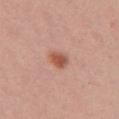Q: Was a biopsy performed?
A: catalogued during a skin exam; not biopsied
Q: What is the anatomic site?
A: the chest
Q: What is the imaging modality?
A: total-body-photography crop, ~15 mm field of view
Q: Automated lesion metrics?
A: a mean CIELAB color near L≈55 a*≈26 b*≈30 and a normalized border contrast of about 8.5
Q: Lesion size?
A: ≈2.5 mm
Q: Patient demographics?
A: female, in their mid- to late 30s
Q: What lighting was used for the tile?
A: white-light